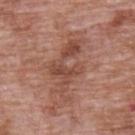  automated_metrics:
    cielab_L: 48
    cielab_a: 22
    cielab_b: 28
    vs_skin_contrast_norm: 6.5
  lighting: white-light
  lesion_size:
    long_diameter_mm_approx: 7.5
  image:
    source: total-body photography crop
    field_of_view_mm: 15
  patient:
    sex: male
    age_approx: 70
  site: upper back